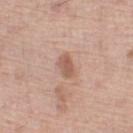Captured during whole-body skin photography for melanoma surveillance; the lesion was not biopsied.
About 3 mm across.
The subject is a male aged approximately 70.
Automated tile analysis of the lesion measured a lesion color around L≈58 a*≈20 b*≈29 in CIELAB, roughly 10 lightness units darker than nearby skin, and a normalized border contrast of about 7.
Located on the left lower leg.
A 15 mm close-up tile from a total-body photography series done for melanoma screening.